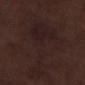Clinical impression:
The lesion was photographed on a routine skin check and not biopsied; there is no pathology result.
Image and clinical context:
A 15 mm close-up tile from a total-body photography series done for melanoma screening. From the right lower leg. A male patient, aged approximately 70.